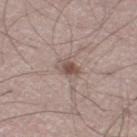follow-up = imaged on a skin check; not biopsied | lighting = white-light illumination | lesion diameter = ≈3 mm | anatomic site = the right thigh | patient = male, aged approximately 50 | image source = total-body-photography crop, ~15 mm field of view.